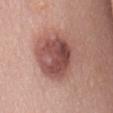biopsy status = imaged on a skin check; not biopsied
subject = female, in their 60s
imaging modality = total-body-photography crop, ~15 mm field of view
anatomic site = the mid back
tile lighting = white-light illumination
lesion size = ~6 mm (longest diameter)